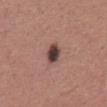notes = total-body-photography surveillance lesion; no biopsy
automated metrics = a nevus-likeness score of about 55/100
acquisition = 15 mm crop, total-body photography
size = about 3.5 mm
patient = male, about 45 years old
site = the abdomen